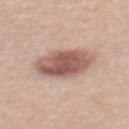Impression:
Part of a total-body skin-imaging series; this lesion was reviewed on a skin check and was not flagged for biopsy.
Clinical summary:
This is a white-light tile. The recorded lesion diameter is about 5.5 mm. Cropped from a whole-body photographic skin survey; the tile spans about 15 mm. The lesion-visualizer software estimated an area of roughly 17 mm², an outline eccentricity of about 0.7 (0 = round, 1 = elongated), and two-axis asymmetry of about 0.15. And it measured a mean CIELAB color near L≈56 a*≈20 b*≈24 and about 15 CIELAB-L* units darker than the surrounding skin. On the mid back. A female patient, roughly 55 years of age.